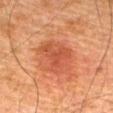Clinical impression: No biopsy was performed on this lesion — it was imaged during a full skin examination and was not determined to be concerning. Acquisition and patient details: The subject is a male roughly 80 years of age. About 5 mm across. Cropped from a total-body skin-imaging series; the visible field is about 15 mm. Automated image analysis of the tile measured a border-irregularity index near 4/10, a color-variation rating of about 3/10, and a peripheral color-asymmetry measure near 1. The tile uses cross-polarized illumination. On the abdomen.